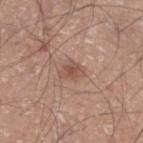Findings:
* follow-up: total-body-photography surveillance lesion; no biopsy
* anatomic site: the leg
* tile lighting: white-light illumination
* TBP lesion metrics: an area of roughly 4.5 mm²; a lesion-to-skin contrast of about 6.5 (normalized; higher = more distinct); an automated nevus-likeness rating near 20 out of 100 and a lesion-detection confidence of about 100/100
* patient: male, about 45 years old
* acquisition: ~15 mm crop, total-body skin-cancer survey